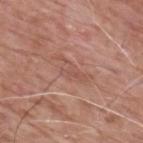  biopsy_status: not biopsied; imaged during a skin examination
  lighting: white-light
  site: upper back
  patient:
    sex: male
    age_approx: 60
  image:
    source: total-body photography crop
    field_of_view_mm: 15
  automated_metrics:
    eccentricity: 0.9
    shape_asymmetry: 0.45
  lesion_size:
    long_diameter_mm_approx: 4.5